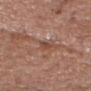The lesion was photographed on a routine skin check and not biopsied; there is no pathology result. The total-body-photography lesion software estimated an average lesion color of about L≈47 a*≈21 b*≈29 (CIELAB), roughly 8 lightness units darker than nearby skin, and a lesion-to-skin contrast of about 6.5 (normalized; higher = more distinct). It also reported a border-irregularity index near 3/10 and radial color variation of about 0. Located on the head or neck. The patient is a male aged around 55. Captured under white-light illumination. Cropped from a whole-body photographic skin survey; the tile spans about 15 mm. The recorded lesion diameter is about 2.5 mm.